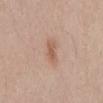Assessment: Imaged during a routine full-body skin examination; the lesion was not biopsied and no histopathology is available. Clinical summary: A female patient in their mid-20s. Imaged with white-light lighting. From the back. The lesion-visualizer software estimated an average lesion color of about L≈58 a*≈20 b*≈30 (CIELAB), a lesion–skin lightness drop of about 8, and a normalized border contrast of about 6.5. The software also gave a nevus-likeness score of about 30/100 and lesion-presence confidence of about 100/100. A 15 mm close-up tile from a total-body photography series done for melanoma screening.